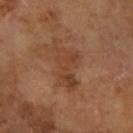This lesion was catalogued during total-body skin photography and was not selected for biopsy. A female subject, aged around 70. The lesion's longest dimension is about 6.5 mm. Cropped from a total-body skin-imaging series; the visible field is about 15 mm. From the arm.